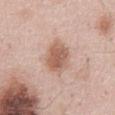Recorded during total-body skin imaging; not selected for excision or biopsy. A 15 mm close-up tile from a total-body photography series done for melanoma screening. This is a white-light tile. Located on the front of the torso. About 5 mm across. A male subject aged around 55.